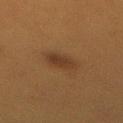Notes:
- follow-up: no biopsy performed (imaged during a skin exam)
- image source: ~15 mm crop, total-body skin-cancer survey
- patient: female, aged 38–42
- site: the back
- tile lighting: cross-polarized illumination
- automated metrics: a mean CIELAB color near L≈29 a*≈15 b*≈26 and a normalized lesion–skin contrast near 7